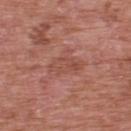{
  "biopsy_status": "not biopsied; imaged during a skin examination",
  "lighting": "white-light",
  "site": "upper back",
  "lesion_size": {
    "long_diameter_mm_approx": 4.0
  },
  "patient": {
    "sex": "male",
    "age_approx": 70
  },
  "image": {
    "source": "total-body photography crop",
    "field_of_view_mm": 15
  }
}